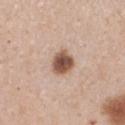follow-up = total-body-photography surveillance lesion; no biopsy
size = ~3.5 mm (longest diameter)
lighting = white-light
subject = male, aged 58 to 62
body site = the front of the torso
imaging modality = ~15 mm crop, total-body skin-cancer survey
automated metrics = two-axis asymmetry of about 0.2